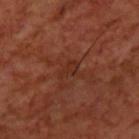Impression: This lesion was catalogued during total-body skin photography and was not selected for biopsy. Image and clinical context: The lesion is on the upper back. The patient is a male aged 68 to 72. The tile uses cross-polarized illumination. Longest diameter approximately 2.5 mm. A 15 mm crop from a total-body photograph taken for skin-cancer surveillance.